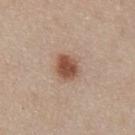This lesion was catalogued during total-body skin photography and was not selected for biopsy.
Cropped from a total-body skin-imaging series; the visible field is about 15 mm.
Captured under white-light illumination.
The patient is a male roughly 55 years of age.
From the abdomen.